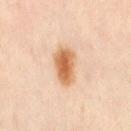Impression: Captured during whole-body skin photography for melanoma surveillance; the lesion was not biopsied. Context: The recorded lesion diameter is about 4.5 mm. A region of skin cropped from a whole-body photographic capture, roughly 15 mm wide. The patient is a female aged 38–42. Captured under cross-polarized illumination. From the right thigh. The lesion-visualizer software estimated a lesion color around L≈66 a*≈22 b*≈39 in CIELAB and a lesion–skin lightness drop of about 14. It also reported a border-irregularity rating of about 1.5/10, a within-lesion color-variation index near 5/10, and peripheral color asymmetry of about 1.5. The software also gave a nevus-likeness score of about 100/100 and lesion-presence confidence of about 100/100.